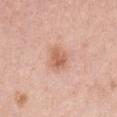Impression: This lesion was catalogued during total-body skin photography and was not selected for biopsy. Background: Measured at roughly 3 mm in maximum diameter. Captured under white-light illumination. A close-up tile cropped from a whole-body skin photograph, about 15 mm across. A female subject aged 38 to 42. From the front of the torso.